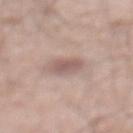The lesion was photographed on a routine skin check and not biopsied; there is no pathology result. The tile uses white-light illumination. A male subject, approximately 70 years of age. Located on the abdomen. Cropped from a whole-body photographic skin survey; the tile spans about 15 mm. About 3 mm across.